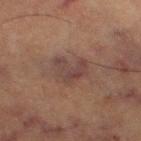Clinical impression: No biopsy was performed on this lesion — it was imaged during a full skin examination and was not determined to be concerning.